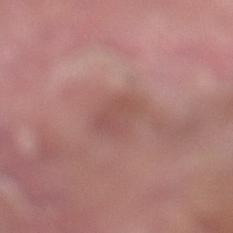| feature | finding |
|---|---|
| subject | male, aged around 40 |
| location | the left lower leg |
| acquisition | ~15 mm tile from a whole-body skin photo |